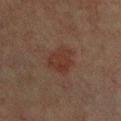Findings:
• subject · male, approximately 60 years of age
• acquisition · 15 mm crop, total-body photography
• site · the chest
• tile lighting · cross-polarized illumination
• automated metrics · a footprint of about 8 mm²; a mean CIELAB color near L≈27 a*≈17 b*≈21, about 5 CIELAB-L* units darker than the surrounding skin, and a lesion-to-skin contrast of about 6.5 (normalized; higher = more distinct); border irregularity of about 3 on a 0–10 scale, a within-lesion color-variation index near 3/10, and a peripheral color-asymmetry measure near 1